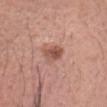notes: no biopsy performed (imaged during a skin exam)
patient: female, aged 63 to 67
image-analysis metrics: an eccentricity of roughly 0.7 and a shape-asymmetry score of about 0.2 (0 = symmetric); a mean CIELAB color near L≈53 a*≈24 b*≈26, about 11 CIELAB-L* units darker than the surrounding skin, and a normalized lesion–skin contrast near 8; a border-irregularity index near 2/10, a within-lesion color-variation index near 4.5/10, and a peripheral color-asymmetry measure near 1.5; a nevus-likeness score of about 80/100 and lesion-presence confidence of about 100/100
location: the head or neck
lighting: white-light
acquisition: 15 mm crop, total-body photography